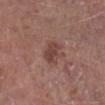Recorded during total-body skin imaging; not selected for excision or biopsy.
The patient is a male in their mid- to late 60s.
The lesion is located on the right lower leg.
About 3 mm across.
The total-body-photography lesion software estimated a shape eccentricity near 0.7 and a symmetry-axis asymmetry near 0.2. The analysis additionally found an average lesion color of about L≈43 a*≈21 b*≈23 (CIELAB) and roughly 9 lightness units darker than nearby skin.
Cropped from a whole-body photographic skin survey; the tile spans about 15 mm.
This is a white-light tile.